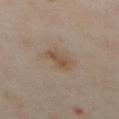{
  "biopsy_status": "not biopsied; imaged during a skin examination",
  "image": {
    "source": "total-body photography crop",
    "field_of_view_mm": 15
  },
  "site": "abdomen",
  "patient": {
    "sex": "male",
    "age_approx": 65
  }
}